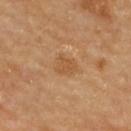Recorded during total-body skin imaging; not selected for excision or biopsy.
This is a cross-polarized tile.
About 2.5 mm across.
A close-up tile cropped from a whole-body skin photograph, about 15 mm across.
The patient is a female aged 68 to 72.
Automated tile analysis of the lesion measured an area of roughly 5 mm². And it measured a lesion color around L≈54 a*≈21 b*≈39 in CIELAB, a lesion–skin lightness drop of about 7, and a normalized lesion–skin contrast near 5.5. The analysis additionally found a border-irregularity index near 2/10, internal color variation of about 1.5 on a 0–10 scale, and peripheral color asymmetry of about 0.5. The analysis additionally found a classifier nevus-likeness of about 5/100 and a lesion-detection confidence of about 100/100.
Located on the upper back.